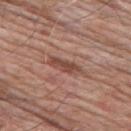biopsy status — catalogued during a skin exam; not biopsied | patient — male, in their 80s | anatomic site — the upper back | image — total-body-photography crop, ~15 mm field of view | illumination — white-light | lesion size — ~4 mm (longest diameter).